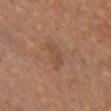- notes — no biopsy performed (imaged during a skin exam)
- imaging modality — total-body-photography crop, ~15 mm field of view
- location — the chest
- patient — female, roughly 55 years of age